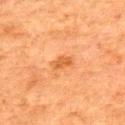No biopsy was performed on this lesion — it was imaged during a full skin examination and was not determined to be concerning. Imaged with cross-polarized lighting. Longest diameter approximately 2.5 mm. A 15 mm close-up tile from a total-body photography series done for melanoma screening. Automated tile analysis of the lesion measured a footprint of about 4 mm², a shape eccentricity near 0.75, and a symmetry-axis asymmetry near 0.3. A male patient aged 78–82. On the upper back.